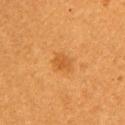follow-up: imaged on a skin check; not biopsied | subject: female, in their mid- to late 50s | imaging modality: ~15 mm crop, total-body skin-cancer survey | illumination: cross-polarized | site: the left upper arm.